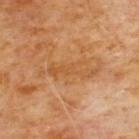notes = imaged on a skin check; not biopsied
size = about 7 mm
body site = the chest
image source = 15 mm crop, total-body photography
patient = male, approximately 60 years of age